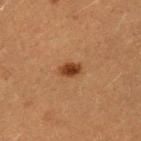| field | value |
|---|---|
| follow-up | total-body-photography surveillance lesion; no biopsy |
| imaging modality | ~15 mm crop, total-body skin-cancer survey |
| patient | male, approximately 40 years of age |
| site | the arm |
| illumination | cross-polarized |